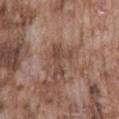Captured during whole-body skin photography for melanoma surveillance; the lesion was not biopsied. A male subject, approximately 75 years of age. From the front of the torso. The lesion's longest dimension is about 3.5 mm. The lesion-visualizer software estimated an area of roughly 5.5 mm² and a symmetry-axis asymmetry near 0.7. The analysis additionally found an average lesion color of about L≈45 a*≈19 b*≈24 (CIELAB), a lesion–skin lightness drop of about 8, and a normalized lesion–skin contrast near 6.5. And it measured border irregularity of about 9 on a 0–10 scale. The analysis additionally found a nevus-likeness score of about 5/100. The tile uses white-light illumination. A 15 mm crop from a total-body photograph taken for skin-cancer surveillance.